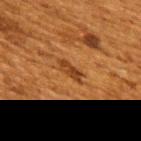Imaged during a routine full-body skin examination; the lesion was not biopsied and no histopathology is available. On the upper back. The subject is a female approximately 50 years of age. The recorded lesion diameter is about 3 mm. Cropped from a total-body skin-imaging series; the visible field is about 15 mm.